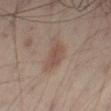{"biopsy_status": "not biopsied; imaged during a skin examination", "patient": {"sex": "male", "age_approx": 50}, "lighting": "cross-polarized", "site": "right thigh", "image": {"source": "total-body photography crop", "field_of_view_mm": 15}, "lesion_size": {"long_diameter_mm_approx": 4.0}}